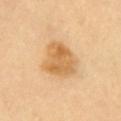workup: catalogued during a skin exam; not biopsied
lesion diameter: about 5 mm
subject: female, about 60 years old
body site: the chest
automated lesion analysis: a border-irregularity index near 2.5/10, internal color variation of about 4.5 on a 0–10 scale, and peripheral color asymmetry of about 1.5
acquisition: ~15 mm crop, total-body skin-cancer survey